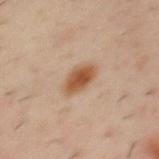Image and clinical context:
On the back. Measured at roughly 3.5 mm in maximum diameter. A male subject aged 38 to 42. Imaged with cross-polarized lighting. A roughly 15 mm field-of-view crop from a total-body skin photograph.